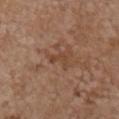  biopsy_status: not biopsied; imaged during a skin examination
  patient:
    sex: female
    age_approx: 65
  site: chest
  image:
    source: total-body photography crop
    field_of_view_mm: 15